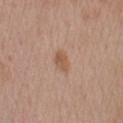| key | value |
|---|---|
| follow-up | total-body-photography surveillance lesion; no biopsy |
| size | ~3 mm (longest diameter) |
| image source | total-body-photography crop, ~15 mm field of view |
| site | the left upper arm |
| illumination | white-light illumination |
| patient | male, roughly 55 years of age |